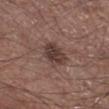  biopsy_status: not biopsied; imaged during a skin examination
  patient:
    sex: male
    age_approx: 55
  lighting: white-light
  image:
    source: total-body photography crop
    field_of_view_mm: 15
  site: right lower leg
  lesion_size:
    long_diameter_mm_approx: 3.5